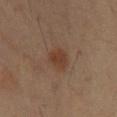Longest diameter approximately 3 mm.
Imaged with cross-polarized lighting.
On the chest.
The subject is a male roughly 60 years of age.
A lesion tile, about 15 mm wide, cut from a 3D total-body photograph.